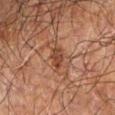Q: Was a biopsy performed?
A: total-body-photography surveillance lesion; no biopsy
Q: What did automated image analysis measure?
A: an area of roughly 4 mm², an outline eccentricity of about 0.8 (0 = round, 1 = elongated), and two-axis asymmetry of about 0.3; border irregularity of about 3 on a 0–10 scale, a color-variation rating of about 2.5/10, and peripheral color asymmetry of about 1; a nevus-likeness score of about 10/100 and lesion-presence confidence of about 100/100
Q: What are the patient's age and sex?
A: male, approximately 70 years of age
Q: Lesion location?
A: the right forearm
Q: How large is the lesion?
A: ≈2.5 mm
Q: What kind of image is this?
A: ~15 mm crop, total-body skin-cancer survey